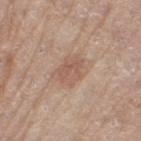workup = no biopsy performed (imaged during a skin exam); body site = the left thigh; acquisition = 15 mm crop, total-body photography; subject = female, roughly 65 years of age; diameter = ≈3.5 mm.